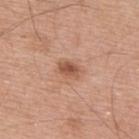biopsy_status: not biopsied; imaged during a skin examination
site: upper back
patient:
  sex: male
  age_approx: 55
automated_metrics:
  area_mm2_approx: 3.5
  border_irregularity_0_10: 2.5
  color_variation_0_10: 2.5
  peripheral_color_asymmetry: 1.0
  nevus_likeness_0_100: 30
  lesion_detection_confidence_0_100: 100
image:
  source: total-body photography crop
  field_of_view_mm: 15
lesion_size:
  long_diameter_mm_approx: 2.5
lighting: white-light